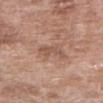Q: Is there a histopathology result?
A: catalogued during a skin exam; not biopsied
Q: What lighting was used for the tile?
A: white-light illumination
Q: What is the lesion's diameter?
A: ~3 mm (longest diameter)
Q: Who is the patient?
A: female, approximately 70 years of age
Q: What kind of image is this?
A: ~15 mm tile from a whole-body skin photo
Q: Where on the body is the lesion?
A: the right upper arm